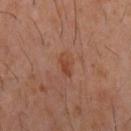Case summary:
• follow-up · no biopsy performed (imaged during a skin exam)
• patient · male, aged around 60
• image source · ~15 mm tile from a whole-body skin photo
• site · the mid back